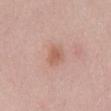<lesion>
  <biopsy_status>not biopsied; imaged during a skin examination</biopsy_status>
  <image>
    <source>total-body photography crop</source>
    <field_of_view_mm>15</field_of_view_mm>
  </image>
  <automated_metrics>
    <area_mm2_approx>4.5</area_mm2_approx>
    <eccentricity>0.75</eccentricity>
    <shape_asymmetry>0.2</shape_asymmetry>
  </automated_metrics>
  <patient>
    <sex>female</sex>
    <age_approx>40</age_approx>
  </patient>
  <site>mid back</site>
  <lesion_size>
    <long_diameter_mm_approx>3.0</long_diameter_mm_approx>
  </lesion_size>
</lesion>